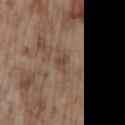This lesion was catalogued during total-body skin photography and was not selected for biopsy. The recorded lesion diameter is about 2.5 mm. Cropped from a total-body skin-imaging series; the visible field is about 15 mm. From the lower back. The tile uses white-light illumination. A male subject, in their mid- to late 70s.